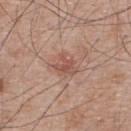- follow-up: total-body-photography surveillance lesion; no biopsy
- lesion diameter: ≈3 mm
- location: the back
- image source: ~15 mm tile from a whole-body skin photo
- subject: male, approximately 65 years of age
- illumination: white-light illumination